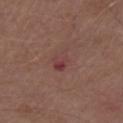Clinical summary: Captured under white-light illumination. From the right upper arm. Measured at roughly 3.5 mm in maximum diameter. A 15 mm crop from a total-body photograph taken for skin-cancer surveillance. A male patient aged 68–72. The total-body-photography lesion software estimated an area of roughly 4.5 mm², an eccentricity of roughly 0.75, and a shape-asymmetry score of about 0.25 (0 = symmetric). The analysis additionally found a border-irregularity rating of about 3/10.